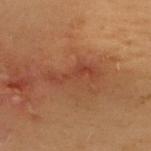Impression: The lesion was photographed on a routine skin check and not biopsied; there is no pathology result. Background: Automated tile analysis of the lesion measured a nevus-likeness score of about 0/100 and a detector confidence of about 100 out of 100 that the crop contains a lesion. From the back. Imaged with cross-polarized lighting. A female patient aged 38–42. A lesion tile, about 15 mm wide, cut from a 3D total-body photograph.